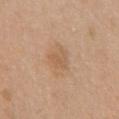No biopsy was performed on this lesion — it was imaged during a full skin examination and was not determined to be concerning. Located on the chest. The total-body-photography lesion software estimated a footprint of about 5 mm², an eccentricity of roughly 0.5, and a shape-asymmetry score of about 0.4 (0 = symmetric). The analysis additionally found a border-irregularity rating of about 3.5/10. The analysis additionally found a nevus-likeness score of about 5/100 and a lesion-detection confidence of about 100/100. About 3 mm across. A female patient aged around 70. A region of skin cropped from a whole-body photographic capture, roughly 15 mm wide. Captured under white-light illumination.